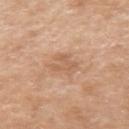workup = total-body-photography surveillance lesion; no biopsy
location = the left upper arm
TBP lesion metrics = a lesion–skin lightness drop of about 7 and a normalized border contrast of about 5; border irregularity of about 5.5 on a 0–10 scale and a color-variation rating of about 1/10
diameter = about 3 mm
tile lighting = white-light
patient = male, aged 58 to 62
image = ~15 mm crop, total-body skin-cancer survey